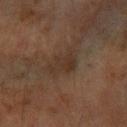notes = catalogued during a skin exam; not biopsied | illumination = cross-polarized illumination | image = total-body-photography crop, ~15 mm field of view | site = the left forearm | subject = female, aged around 70 | lesion diameter = ≈4 mm.